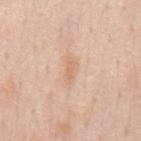Recorded during total-body skin imaging; not selected for excision or biopsy. A close-up tile cropped from a whole-body skin photograph, about 15 mm across. The lesion-visualizer software estimated a classifier nevus-likeness of about 0/100 and a detector confidence of about 100 out of 100 that the crop contains a lesion. The lesion's longest dimension is about 3.5 mm. Imaged with white-light lighting. The patient is a male in their 60s. Located on the mid back.